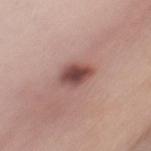The lesion was photographed on a routine skin check and not biopsied; there is no pathology result. The total-body-photography lesion software estimated an area of roughly 7 mm² and a shape-asymmetry score of about 0.2 (0 = symmetric). The software also gave an average lesion color of about L≈47 a*≈23 b*≈22 (CIELAB) and a normalized lesion–skin contrast near 10.5. The analysis additionally found a peripheral color-asymmetry measure near 1.5. This is a white-light tile. From the chest. A female patient, approximately 45 years of age. A roughly 15 mm field-of-view crop from a total-body skin photograph. The lesion's longest dimension is about 4 mm.